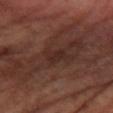biopsy status — total-body-photography surveillance lesion; no biopsy | imaging modality — total-body-photography crop, ~15 mm field of view | tile lighting — cross-polarized | location — the right forearm | patient — female, aged 63 to 67.